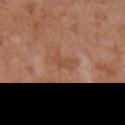This lesion was catalogued during total-body skin photography and was not selected for biopsy.
Imaged with cross-polarized lighting.
An algorithmic analysis of the crop reported a border-irregularity index near 4.5/10 and internal color variation of about 0.5 on a 0–10 scale.
Approximately 3 mm at its widest.
From the right upper arm.
The patient is a male approximately 55 years of age.
A close-up tile cropped from a whole-body skin photograph, about 15 mm across.